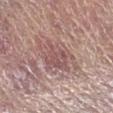Cropped from a total-body skin-imaging series; the visible field is about 15 mm. On the right forearm. The subject is a male in their mid- to late 60s.